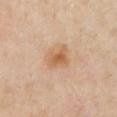Impression: The lesion was tiled from a total-body skin photograph and was not biopsied. Context: The subject is a female approximately 40 years of age. Imaged with cross-polarized lighting. On the chest. The lesion's longest dimension is about 3 mm. A region of skin cropped from a whole-body photographic capture, roughly 15 mm wide. An algorithmic analysis of the crop reported an outline eccentricity of about 0.6 (0 = round, 1 = elongated). The software also gave about 9 CIELAB-L* units darker than the surrounding skin.